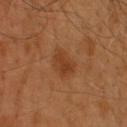biopsy status — imaged on a skin check; not biopsied
illumination — cross-polarized illumination
image — ~15 mm tile from a whole-body skin photo
patient — male, aged around 40
location — the upper back